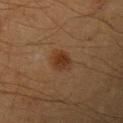  site: arm
  automated_metrics:
    cielab_L: 31
    cielab_a: 18
    cielab_b: 28
    vs_skin_darker_L: 8.0
    nevus_likeness_0_100: 100
    lesion_detection_confidence_0_100: 100
  lesion_size:
    long_diameter_mm_approx: 3.0
  patient:
    sex: male
    age_approx: 40
  image:
    source: total-body photography crop
    field_of_view_mm: 15
  lighting: cross-polarized